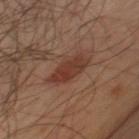| key | value |
|---|---|
| notes | catalogued during a skin exam; not biopsied |
| body site | the chest |
| subject | male, aged 63–67 |
| image source | total-body-photography crop, ~15 mm field of view |